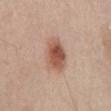| key | value |
|---|---|
| biopsy status | no biopsy performed (imaged during a skin exam) |